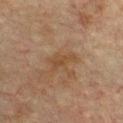Imaged during a routine full-body skin examination; the lesion was not biopsied and no histopathology is available.
Located on the front of the torso.
The tile uses cross-polarized illumination.
A 15 mm close-up extracted from a 3D total-body photography capture.
A male subject, aged approximately 75.
The lesion's longest dimension is about 3.5 mm.